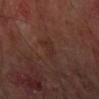Q: Was a biopsy performed?
A: total-body-photography surveillance lesion; no biopsy
Q: How large is the lesion?
A: ≈4 mm
Q: Who is the patient?
A: male, approximately 60 years of age
Q: How was the tile lit?
A: cross-polarized
Q: What kind of image is this?
A: total-body-photography crop, ~15 mm field of view
Q: Lesion location?
A: the arm